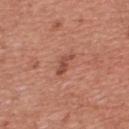Notes:
• notes · catalogued during a skin exam; not biopsied
• lesion size · about 3 mm
• automated metrics · a border-irregularity index near 4.5/10, a color-variation rating of about 0.5/10, and a peripheral color-asymmetry measure near 0
• patient · male, in their mid- to late 40s
• lighting · white-light illumination
• anatomic site · the chest
• acquisition · ~15 mm crop, total-body skin-cancer survey